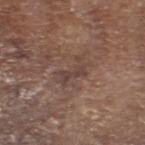workup: imaged on a skin check; not biopsied